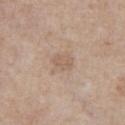Case summary:
* workup · catalogued during a skin exam; not biopsied
* lighting · white-light illumination
* body site · the chest
* image source · total-body-photography crop, ~15 mm field of view
* subject · male, roughly 65 years of age
* TBP lesion metrics · an area of roughly 5 mm², an outline eccentricity of about 0.45 (0 = round, 1 = elongated), and two-axis asymmetry of about 0.3; an automated nevus-likeness rating near 0 out of 100 and lesion-presence confidence of about 100/100
* size · ≈2.5 mm